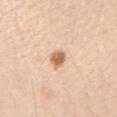notes: total-body-photography surveillance lesion; no biopsy
diameter: ≈2.5 mm
automated metrics: an area of roughly 4 mm², an outline eccentricity of about 0.65 (0 = round, 1 = elongated), and a shape-asymmetry score of about 0.25 (0 = symmetric); a mean CIELAB color near L≈66 a*≈21 b*≈35, a lesion–skin lightness drop of about 15, and a lesion-to-skin contrast of about 9 (normalized; higher = more distinct)
site: the right upper arm
acquisition: ~15 mm crop, total-body skin-cancer survey
illumination: white-light
subject: female, approximately 25 years of age